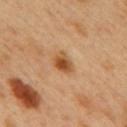Assessment:
Captured during whole-body skin photography for melanoma surveillance; the lesion was not biopsied.
Clinical summary:
A lesion tile, about 15 mm wide, cut from a 3D total-body photograph. Measured at roughly 3 mm in maximum diameter. On the mid back. A male patient, in their 50s.